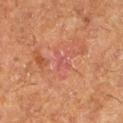Part of a total-body skin-imaging series; this lesion was reviewed on a skin check and was not flagged for biopsy. The lesion is on the leg. The subject is a female approximately 50 years of age. A 15 mm close-up extracted from a 3D total-body photography capture. Approximately 3 mm at its widest. Imaged with cross-polarized lighting.